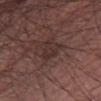  biopsy_status: not biopsied; imaged during a skin examination
  patient:
    sex: male
    age_approx: 60
  lighting: white-light
  lesion_size:
    long_diameter_mm_approx: 3.0
  image:
    source: total-body photography crop
    field_of_view_mm: 15
  site: right lower leg
  automated_metrics:
    area_mm2_approx: 5.0
    eccentricity: 0.55
    cielab_L: 31
    cielab_a: 17
    cielab_b: 18
    vs_skin_darker_L: 5.0
    vs_skin_contrast_norm: 5.5
    nevus_likeness_0_100: 0
    lesion_detection_confidence_0_100: 85